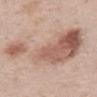Impression:
Captured during whole-body skin photography for melanoma surveillance; the lesion was not biopsied.
Context:
A region of skin cropped from a whole-body photographic capture, roughly 15 mm wide. The lesion's longest dimension is about 11 mm. A male patient approximately 60 years of age. The total-body-photography lesion software estimated a lesion color around L≈61 a*≈18 b*≈26 in CIELAB and a normalized lesion–skin contrast near 6.5. The lesion is on the abdomen.